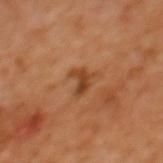Clinical impression: Recorded during total-body skin imaging; not selected for excision or biopsy. Context: Measured at roughly 3 mm in maximum diameter. A region of skin cropped from a whole-body photographic capture, roughly 15 mm wide. The subject is a female aged approximately 40. The tile uses cross-polarized illumination. The total-body-photography lesion software estimated a shape-asymmetry score of about 0.55 (0 = symmetric). And it measured a lesion–skin lightness drop of about 10 and a lesion-to-skin contrast of about 8 (normalized; higher = more distinct). And it measured a detector confidence of about 100 out of 100 that the crop contains a lesion. The lesion is located on the upper back.